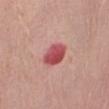A female patient, aged around 65. About 3.5 mm across. The lesion is on the right thigh. Captured under white-light illumination. A 15 mm close-up tile from a total-body photography series done for melanoma screening. Automated image analysis of the tile measured about 15 CIELAB-L* units darker than the surrounding skin and a lesion-to-skin contrast of about 10 (normalized; higher = more distinct).